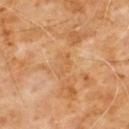Clinical summary:
A 15 mm crop from a total-body photograph taken for skin-cancer surveillance. The tile uses cross-polarized illumination. A male subject approximately 60 years of age. The lesion is on the chest. The lesion's longest dimension is about 3 mm.